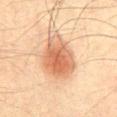Part of a total-body skin-imaging series; this lesion was reviewed on a skin check and was not flagged for biopsy. A close-up tile cropped from a whole-body skin photograph, about 15 mm across. The lesion's longest dimension is about 5.5 mm. Automated tile analysis of the lesion measured an eccentricity of roughly 0.65 and a symmetry-axis asymmetry near 0.15. The software also gave an average lesion color of about L≈52 a*≈20 b*≈31 (CIELAB) and a lesion-to-skin contrast of about 8 (normalized; higher = more distinct). It also reported border irregularity of about 2 on a 0–10 scale and a within-lesion color-variation index near 5/10. From the abdomen. A male subject, in their mid- to late 60s. The tile uses cross-polarized illumination.